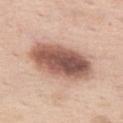Q: Is there a histopathology result?
A: imaged on a skin check; not biopsied
Q: Lesion size?
A: about 8 mm
Q: Lesion location?
A: the left thigh
Q: How was the tile lit?
A: white-light illumination
Q: What is the imaging modality?
A: total-body-photography crop, ~15 mm field of view
Q: Automated lesion metrics?
A: an average lesion color of about L≈56 a*≈20 b*≈27 (CIELAB), roughly 18 lightness units darker than nearby skin, and a normalized lesion–skin contrast near 11; an automated nevus-likeness rating near 95 out of 100 and a detector confidence of about 100 out of 100 that the crop contains a lesion
Q: Patient demographics?
A: female, approximately 55 years of age